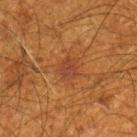The lesion was tiled from a total-body skin photograph and was not biopsied.
This is a cross-polarized tile.
A male patient, aged 58 to 62.
A lesion tile, about 15 mm wide, cut from a 3D total-body photograph.
On the right lower leg.
Approximately 2.5 mm at its widest.